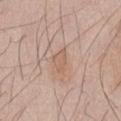biopsy status: imaged on a skin check; not biopsied | patient: male, aged 43–47 | site: the mid back | image: ~15 mm tile from a whole-body skin photo | illumination: white-light illumination | TBP lesion metrics: a footprint of about 4 mm² and an outline eccentricity of about 0.75 (0 = round, 1 = elongated); radial color variation of about 0.5; an automated nevus-likeness rating near 5 out of 100 and lesion-presence confidence of about 100/100.